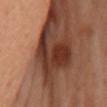notes: total-body-photography surveillance lesion; no biopsy
anatomic site: the left arm
automated lesion analysis: an outline eccentricity of about 0.65 (0 = round, 1 = elongated) and a symmetry-axis asymmetry near 0.45; a mean CIELAB color near L≈29 a*≈21 b*≈24, roughly 13 lightness units darker than nearby skin, and a lesion-to-skin contrast of about 12 (normalized; higher = more distinct); a detector confidence of about 70 out of 100 that the crop contains a lesion
patient: female, aged 58 to 62
illumination: cross-polarized
size: about 7 mm
image: 15 mm crop, total-body photography